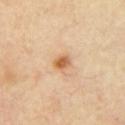Impression:
The lesion was tiled from a total-body skin photograph and was not biopsied.
Clinical summary:
Located on the chest. The recorded lesion diameter is about 2 mm. The patient is a male about 55 years old. A region of skin cropped from a whole-body photographic capture, roughly 15 mm wide. Automated image analysis of the tile measured a footprint of about 3.5 mm² and an outline eccentricity of about 0.6 (0 = round, 1 = elongated). It also reported a lesion color around L≈63 a*≈22 b*≈40 in CIELAB, roughly 12 lightness units darker than nearby skin, and a lesion-to-skin contrast of about 8.5 (normalized; higher = more distinct). The analysis additionally found a border-irregularity rating of about 2/10 and radial color variation of about 1.5.